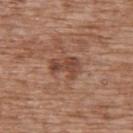Q: Was a biopsy performed?
A: total-body-photography surveillance lesion; no biopsy
Q: Illumination type?
A: white-light illumination
Q: Patient demographics?
A: female, approximately 75 years of age
Q: What is the anatomic site?
A: the upper back
Q: How was this image acquired?
A: ~15 mm crop, total-body skin-cancer survey
Q: What is the lesion's diameter?
A: ≈3.5 mm